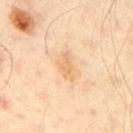The lesion was photographed on a routine skin check and not biopsied; there is no pathology result.
Imaged with cross-polarized lighting.
A male patient aged approximately 50.
On the arm.
The lesion's longest dimension is about 3 mm.
An algorithmic analysis of the crop reported a footprint of about 4.5 mm², an outline eccentricity of about 0.85 (0 = round, 1 = elongated), and a shape-asymmetry score of about 0.35 (0 = symmetric). The analysis additionally found a mean CIELAB color near L≈73 a*≈19 b*≈40. The analysis additionally found a border-irregularity rating of about 3.5/10 and a peripheral color-asymmetry measure near 0.5.
Cropped from a whole-body photographic skin survey; the tile spans about 15 mm.